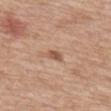Assessment: No biopsy was performed on this lesion — it was imaged during a full skin examination and was not determined to be concerning. Background: Located on the mid back. Measured at roughly 2.5 mm in maximum diameter. This image is a 15 mm lesion crop taken from a total-body photograph. The patient is a male roughly 80 years of age.